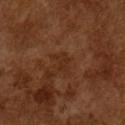Impression: The lesion was photographed on a routine skin check and not biopsied; there is no pathology result. Clinical summary: A male subject aged 63–67. A 15 mm close-up extracted from a 3D total-body photography capture. This is a cross-polarized tile. Approximately 3 mm at its widest.